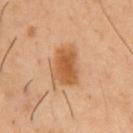Impression:
Captured during whole-body skin photography for melanoma surveillance; the lesion was not biopsied.
Image and clinical context:
A male patient about 50 years old. A lesion tile, about 15 mm wide, cut from a 3D total-body photograph. On the chest.